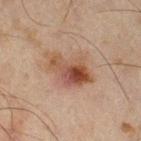Assessment:
The lesion was tiled from a total-body skin photograph and was not biopsied.
Context:
Located on the right thigh. Cropped from a whole-body photographic skin survey; the tile spans about 15 mm. A male subject roughly 45 years of age. An algorithmic analysis of the crop reported a lesion area of about 13 mm² and a shape eccentricity near 0.75. It also reported a lesion color around L≈40 a*≈18 b*≈25 in CIELAB and a normalized border contrast of about 8.5. The analysis additionally found a border-irregularity index near 3/10, a color-variation rating of about 9.5/10, and peripheral color asymmetry of about 3.5. The tile uses cross-polarized illumination. Measured at roughly 5 mm in maximum diameter.